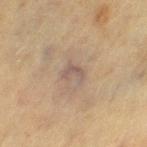<record>
  <biopsy_status>not biopsied; imaged during a skin examination</biopsy_status>
  <lesion_size>
    <long_diameter_mm_approx>2.5</long_diameter_mm_approx>
  </lesion_size>
  <lighting>cross-polarized</lighting>
  <automated_metrics>
    <area_mm2_approx>4.0</area_mm2_approx>
    <eccentricity>0.7</eccentricity>
    <shape_asymmetry>0.45</shape_asymmetry>
    <vs_skin_darker_L>6.0</vs_skin_darker_L>
    <vs_skin_contrast_norm>6.5</vs_skin_contrast_norm>
    <border_irregularity_0_10>4.5</border_irregularity_0_10>
    <color_variation_0_10>1.0</color_variation_0_10>
    <peripheral_color_asymmetry>0.0</peripheral_color_asymmetry>
    <nevus_likeness_0_100>0</nevus_likeness_0_100>
    <lesion_detection_confidence_0_100>75</lesion_detection_confidence_0_100>
  </automated_metrics>
  <site>leg</site>
  <image>
    <source>total-body photography crop</source>
    <field_of_view_mm>15</field_of_view_mm>
  </image>
  <patient>
    <sex>female</sex>
    <age_approx>55</age_approx>
  </patient>
</record>